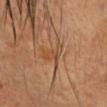Captured during whole-body skin photography for melanoma surveillance; the lesion was not biopsied. This is a cross-polarized tile. From the head or neck. A 15 mm close-up tile from a total-body photography series done for melanoma screening. A female subject, about 55 years old. Longest diameter approximately 3.5 mm.